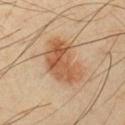The lesion was tiled from a total-body skin photograph and was not biopsied.
The lesion is on the chest.
The patient is a male aged 38–42.
Cropped from a whole-body photographic skin survey; the tile spans about 15 mm.